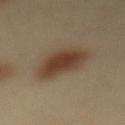{
  "biopsy_status": "not biopsied; imaged during a skin examination",
  "site": "mid back",
  "image": {
    "source": "total-body photography crop",
    "field_of_view_mm": 15
  },
  "patient": {
    "sex": "female",
    "age_approx": 40
  },
  "lesion_size": {
    "long_diameter_mm_approx": 7.0
  },
  "lighting": "cross-polarized"
}